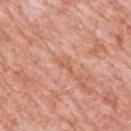Recorded during total-body skin imaging; not selected for excision or biopsy.
About 3 mm across.
The total-body-photography lesion software estimated roughly 6 lightness units darker than nearby skin and a normalized lesion–skin contrast near 5. The analysis additionally found a classifier nevus-likeness of about 0/100 and a lesion-detection confidence of about 60/100.
Located on the back.
This is a white-light tile.
A 15 mm close-up tile from a total-body photography series done for melanoma screening.
A male subject, aged 78 to 82.